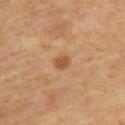Notes:
* notes — total-body-photography surveillance lesion; no biopsy
* site — the back
* lighting — cross-polarized
* patient — male, approximately 55 years of age
* image source — total-body-photography crop, ~15 mm field of view